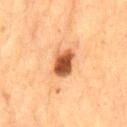The lesion was photographed on a routine skin check and not biopsied; there is no pathology result.